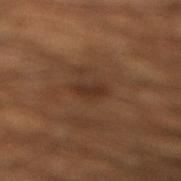An algorithmic analysis of the crop reported an outline eccentricity of about 0.8 (0 = round, 1 = elongated) and a symmetry-axis asymmetry near 0.3. The software also gave a mean CIELAB color near L≈29 a*≈18 b*≈26 and a lesion-to-skin contrast of about 6.5 (normalized; higher = more distinct).
About 2.5 mm across.
This is a cross-polarized tile.
A region of skin cropped from a whole-body photographic capture, roughly 15 mm wide.
The patient is a male roughly 45 years of age.
The lesion is located on the right lower leg.